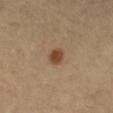Case summary:
* location: the left leg
* image: total-body-photography crop, ~15 mm field of view
* illumination: cross-polarized illumination
* patient: female, aged approximately 60